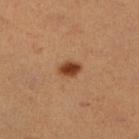No biopsy was performed on this lesion — it was imaged during a full skin examination and was not determined to be concerning.
About 2.5 mm across.
A 15 mm crop from a total-body photograph taken for skin-cancer surveillance.
The lesion-visualizer software estimated a border-irregularity index near 1.5/10 and internal color variation of about 4 on a 0–10 scale. And it measured an automated nevus-likeness rating near 100 out of 100 and a detector confidence of about 100 out of 100 that the crop contains a lesion.
Imaged with cross-polarized lighting.
On the right lower leg.
The patient is a female roughly 55 years of age.